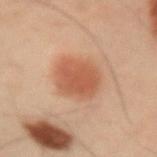Q: What did automated image analysis measure?
A: a shape eccentricity near 0.65 and a symmetry-axis asymmetry near 0.15; a border-irregularity index near 2/10, a within-lesion color-variation index near 2.5/10, and peripheral color asymmetry of about 0.5; an automated nevus-likeness rating near 100 out of 100 and a lesion-detection confidence of about 100/100
Q: What lighting was used for the tile?
A: cross-polarized illumination
Q: What is the imaging modality?
A: ~15 mm tile from a whole-body skin photo
Q: How large is the lesion?
A: about 5.5 mm
Q: Patient demographics?
A: male, approximately 55 years of age
Q: Lesion location?
A: the left upper arm